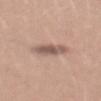Q: Was a biopsy performed?
A: imaged on a skin check; not biopsied
Q: Illumination type?
A: white-light
Q: Automated lesion metrics?
A: a border-irregularity rating of about 3/10, internal color variation of about 4 on a 0–10 scale, and peripheral color asymmetry of about 1; a classifier nevus-likeness of about 10/100
Q: What kind of image is this?
A: ~15 mm tile from a whole-body skin photo
Q: Who is the patient?
A: male, aged 28–32
Q: How large is the lesion?
A: ≈4.5 mm
Q: Where on the body is the lesion?
A: the mid back